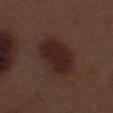{"biopsy_status": "not biopsied; imaged during a skin examination", "patient": {"sex": "male", "age_approx": 70}, "site": "left thigh", "image": {"source": "total-body photography crop", "field_of_view_mm": 15}}